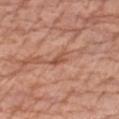biopsy status: catalogued during a skin exam; not biopsied
imaging modality: ~15 mm tile from a whole-body skin photo
illumination: white-light
subject: male, roughly 75 years of age
anatomic site: the left forearm
size: ~2.5 mm (longest diameter)